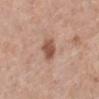workup: no biopsy performed (imaged during a skin exam)
body site: the left lower leg
acquisition: ~15 mm crop, total-body skin-cancer survey
tile lighting: white-light illumination
image-analysis metrics: a footprint of about 6 mm², an outline eccentricity of about 0.5 (0 = round, 1 = elongated), and two-axis asymmetry of about 0.15; about 12 CIELAB-L* units darker than the surrounding skin and a lesion-to-skin contrast of about 8.5 (normalized; higher = more distinct); a nevus-likeness score of about 85/100 and a lesion-detection confidence of about 100/100
diameter: ≈3 mm
subject: female, in their mid-60s